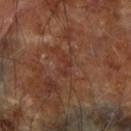| feature | finding |
|---|---|
| anatomic site | the right forearm |
| acquisition | total-body-photography crop, ~15 mm field of view |
| subject | male, in their mid- to late 60s |